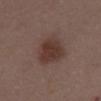Clinical summary:
Automated image analysis of the tile measured a footprint of about 13 mm², an outline eccentricity of about 0.6 (0 = round, 1 = elongated), and two-axis asymmetry of about 0.2. The analysis additionally found about 9 CIELAB-L* units darker than the surrounding skin and a normalized lesion–skin contrast near 8.5. The software also gave a classifier nevus-likeness of about 95/100 and a lesion-detection confidence of about 100/100. The lesion is on the abdomen. Longest diameter approximately 4.5 mm. Cropped from a total-body skin-imaging series; the visible field is about 15 mm. Imaged with white-light lighting. A male subject approximately 50 years of age.